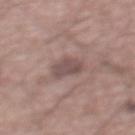Q: Was this lesion biopsied?
A: total-body-photography surveillance lesion; no biopsy
Q: Where on the body is the lesion?
A: the mid back
Q: What is the imaging modality?
A: ~15 mm tile from a whole-body skin photo
Q: Who is the patient?
A: male, aged 53–57